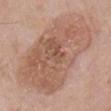Case summary:
– follow-up · imaged on a skin check; not biopsied
– diameter · ≈12 mm
– site · the chest
– subject · male, aged 58–62
– image source · total-body-photography crop, ~15 mm field of view
– tile lighting · white-light illumination